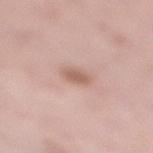| field | value |
|---|---|
| body site | the right lower leg |
| lesion size | ≈2.5 mm |
| patient | female, approximately 50 years of age |
| image-analysis metrics | a lesion color around L≈61 a*≈20 b*≈26 in CIELAB, about 10 CIELAB-L* units darker than the surrounding skin, and a normalized border contrast of about 6.5; a border-irregularity index near 1.5/10, internal color variation of about 1.5 on a 0–10 scale, and peripheral color asymmetry of about 0.5 |
| lighting | white-light |
| imaging modality | 15 mm crop, total-body photography |